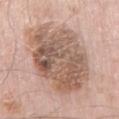Notes:
- follow-up: total-body-photography surveillance lesion; no biopsy